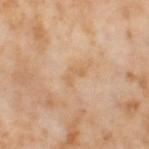The lesion was tiled from a total-body skin photograph and was not biopsied. A 15 mm crop from a total-body photograph taken for skin-cancer surveillance. Longest diameter approximately 2.5 mm. A female subject, in their mid- to late 50s. Imaged with cross-polarized lighting. The lesion is located on the left thigh.